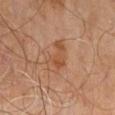* biopsy status · catalogued during a skin exam; not biopsied